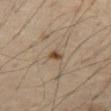Automated image analysis of the tile measured a footprint of about 2.5 mm², a shape eccentricity near 0.8, and a symmetry-axis asymmetry near 0.4. The software also gave a lesion–skin lightness drop of about 11 and a normalized lesion–skin contrast near 9. It also reported an automated nevus-likeness rating near 90 out of 100 and a lesion-detection confidence of about 100/100. The tile uses cross-polarized illumination. Located on the abdomen. Longest diameter approximately 2 mm. A 15 mm close-up tile from a total-body photography series done for melanoma screening. A male subject in their 70s.